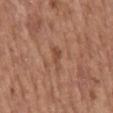Recorded during total-body skin imaging; not selected for excision or biopsy.
The tile uses white-light illumination.
A roughly 15 mm field-of-view crop from a total-body skin photograph.
An algorithmic analysis of the crop reported a lesion area of about 3 mm², an eccentricity of roughly 0.85, and two-axis asymmetry of about 0.5. And it measured a border-irregularity rating of about 5/10 and internal color variation of about 1 on a 0–10 scale. It also reported an automated nevus-likeness rating near 0 out of 100 and a detector confidence of about 100 out of 100 that the crop contains a lesion.
On the mid back.
Approximately 2.5 mm at its widest.
A female subject approximately 65 years of age.